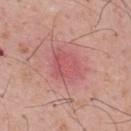{
  "biopsy_status": "not biopsied; imaged during a skin examination",
  "image": {
    "source": "total-body photography crop",
    "field_of_view_mm": 15
  },
  "site": "upper back",
  "lighting": "white-light",
  "patient": {
    "sex": "male",
    "age_approx": 55
  }
}